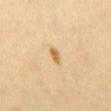Part of a total-body skin-imaging series; this lesion was reviewed on a skin check and was not flagged for biopsy.
Measured at roughly 2.5 mm in maximum diameter.
The lesion-visualizer software estimated a footprint of about 2.5 mm² and an eccentricity of roughly 0.85. The software also gave a lesion color around L≈65 a*≈18 b*≈43 in CIELAB and a lesion-to-skin contrast of about 8 (normalized; higher = more distinct). It also reported border irregularity of about 3 on a 0–10 scale, a color-variation rating of about 1/10, and radial color variation of about 0. It also reported a nevus-likeness score of about 90/100 and lesion-presence confidence of about 100/100.
This image is a 15 mm lesion crop taken from a total-body photograph.
A male subject approximately 45 years of age.
From the abdomen.